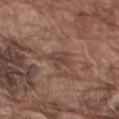Q: Is there a histopathology result?
A: total-body-photography surveillance lesion; no biopsy
Q: What is the lesion's diameter?
A: ≈2.5 mm
Q: How was the tile lit?
A: white-light
Q: Patient demographics?
A: male, roughly 75 years of age
Q: What kind of image is this?
A: ~15 mm crop, total-body skin-cancer survey
Q: What is the anatomic site?
A: the right upper arm
Q: Automated lesion metrics?
A: border irregularity of about 4 on a 0–10 scale, a within-lesion color-variation index near 2.5/10, and radial color variation of about 1